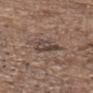Notes:
- workup — no biopsy performed (imaged during a skin exam)
- site — the head or neck
- patient — male, aged 78–82
- acquisition — ~15 mm tile from a whole-body skin photo
- automated metrics — a footprint of about 6.5 mm² and a shape-asymmetry score of about 0.35 (0 = symmetric); border irregularity of about 3.5 on a 0–10 scale, a within-lesion color-variation index near 6.5/10, and a peripheral color-asymmetry measure near 2.5; a classifier nevus-likeness of about 0/100 and a detector confidence of about 90 out of 100 that the crop contains a lesion
- lighting — white-light illumination
- size — about 3 mm